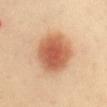Clinical impression: Recorded during total-body skin imaging; not selected for excision or biopsy. Image and clinical context: A lesion tile, about 15 mm wide, cut from a 3D total-body photograph. A female patient about 40 years old. On the abdomen. The lesion-visualizer software estimated an average lesion color of about L≈62 a*≈25 b*≈37 (CIELAB), roughly 15 lightness units darker than nearby skin, and a lesion-to-skin contrast of about 9 (normalized; higher = more distinct). It also reported border irregularity of about 1 on a 0–10 scale and radial color variation of about 1. The software also gave an automated nevus-likeness rating near 100 out of 100. Approximately 6 mm at its widest. Captured under cross-polarized illumination.